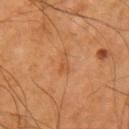The lesion was tiled from a total-body skin photograph and was not biopsied. The patient is a male in their 70s. The tile uses cross-polarized illumination. Longest diameter approximately 2.5 mm. A 15 mm close-up tile from a total-body photography series done for melanoma screening. On the right upper arm.